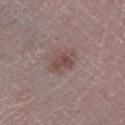Captured during whole-body skin photography for melanoma surveillance; the lesion was not biopsied. Longest diameter approximately 2.5 mm. On the left lower leg. A male patient aged approximately 75. A lesion tile, about 15 mm wide, cut from a 3D total-body photograph.